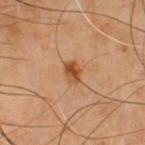| feature | finding |
|---|---|
| follow-up | imaged on a skin check; not biopsied |
| anatomic site | the front of the torso |
| image source | ~15 mm crop, total-body skin-cancer survey |
| subject | male, aged around 55 |